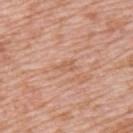notes — catalogued during a skin exam; not biopsied
image — 15 mm crop, total-body photography
patient — male, aged around 50
site — the left upper arm
lighting — white-light
diameter — ~3 mm (longest diameter)